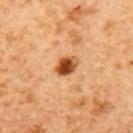{"biopsy_status": "not biopsied; imaged during a skin examination", "image": {"source": "total-body photography crop", "field_of_view_mm": 15}, "site": "upper back", "patient": {"sex": "female", "age_approx": 55}}